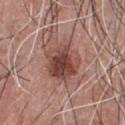Findings:
* biopsy status · total-body-photography surveillance lesion; no biopsy
* image · ~15 mm crop, total-body skin-cancer survey
* illumination · white-light illumination
* body site · the chest
* size · about 5 mm
* subject · male, aged 43–47